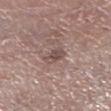This lesion was catalogued during total-body skin photography and was not selected for biopsy. Imaged with white-light lighting. On the right lower leg. The lesion's longest dimension is about 2.5 mm. A 15 mm close-up extracted from a 3D total-body photography capture. A male subject roughly 55 years of age.